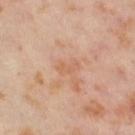<record>
<biopsy_status>not biopsied; imaged during a skin examination</biopsy_status>
<image>
  <source>total-body photography crop</source>
  <field_of_view_mm>15</field_of_view_mm>
</image>
<site>left thigh</site>
<patient>
  <sex>female</sex>
  <age_approx>55</age_approx>
</patient>
</record>